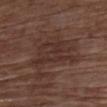This lesion was catalogued during total-body skin photography and was not selected for biopsy.